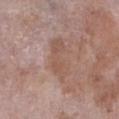No biopsy was performed on this lesion — it was imaged during a full skin examination and was not determined to be concerning.
About 5.5 mm across.
On the left lower leg.
A female subject aged around 70.
Cropped from a whole-body photographic skin survey; the tile spans about 15 mm.
The total-body-photography lesion software estimated a footprint of about 10 mm², an eccentricity of roughly 0.9, and a shape-asymmetry score of about 0.35 (0 = symmetric). And it measured a color-variation rating of about 2.5/10 and radial color variation of about 0.5. The software also gave a nevus-likeness score of about 0/100 and a detector confidence of about 100 out of 100 that the crop contains a lesion.